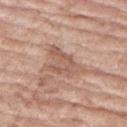Notes:
– workup · catalogued during a skin exam; not biopsied
– location · the right upper arm
– diameter · ≈5 mm
– subject · female, approximately 75 years of age
– automated lesion analysis · a symmetry-axis asymmetry near 0.45; a mean CIELAB color near L≈57 a*≈20 b*≈27, a lesion–skin lightness drop of about 9, and a normalized border contrast of about 6; internal color variation of about 3.5 on a 0–10 scale and a peripheral color-asymmetry measure near 1
– image source · ~15 mm crop, total-body skin-cancer survey